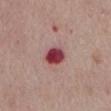Clinical impression:
Recorded during total-body skin imaging; not selected for excision or biopsy.
Background:
The lesion-visualizer software estimated an average lesion color of about L≈43 a*≈33 b*≈20 (CIELAB) and about 18 CIELAB-L* units darker than the surrounding skin. And it measured border irregularity of about 1.5 on a 0–10 scale, a within-lesion color-variation index near 6/10, and peripheral color asymmetry of about 2. A 15 mm close-up tile from a total-body photography series done for melanoma screening. Approximately 3 mm at its widest. On the mid back. Captured under white-light illumination. A male patient in their mid-70s.